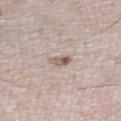biopsy status = imaged on a skin check; not biopsied
subject = male, in their mid-70s
imaging modality = ~15 mm tile from a whole-body skin photo
illumination = white-light
lesion size = ~2.5 mm (longest diameter)
anatomic site = the right lower leg